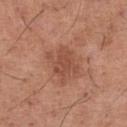<record>
<biopsy_status>not biopsied; imaged during a skin examination</biopsy_status>
<site>left thigh</site>
<lighting>white-light</lighting>
<lesion_size>
  <long_diameter_mm_approx>4.0</long_diameter_mm_approx>
</lesion_size>
<automated_metrics>
  <area_mm2_approx>9.5</area_mm2_approx>
  <eccentricity>0.55</eccentricity>
  <shape_asymmetry>0.35</shape_asymmetry>
</automated_metrics>
<patient>
  <sex>male</sex>
  <age_approx>65</age_approx>
</patient>
<image>
  <source>total-body photography crop</source>
  <field_of_view_mm>15</field_of_view_mm>
</image>
</record>